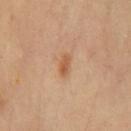follow-up — total-body-photography surveillance lesion; no biopsy
lesion size — about 2.5 mm
image — ~15 mm crop, total-body skin-cancer survey
body site — the chest
tile lighting — cross-polarized illumination